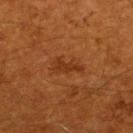Recorded during total-body skin imaging; not selected for excision or biopsy.